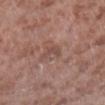follow-up: imaged on a skin check; not biopsied
location: the leg
acquisition: ~15 mm crop, total-body skin-cancer survey
tile lighting: white-light
patient: male, aged around 55